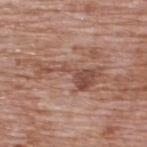Clinical impression:
Part of a total-body skin-imaging series; this lesion was reviewed on a skin check and was not flagged for biopsy.
Background:
The lesion is located on the upper back. An algorithmic analysis of the crop reported an area of roughly 11 mm², an outline eccentricity of about 0.95 (0 = round, 1 = elongated), and a shape-asymmetry score of about 0.7 (0 = symmetric). The analysis additionally found an average lesion color of about L≈49 a*≈22 b*≈27 (CIELAB). A 15 mm close-up tile from a total-body photography series done for melanoma screening. A male subject, aged 68–72. The lesion's longest dimension is about 7 mm.